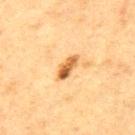* notes: no biopsy performed (imaged during a skin exam)
* lesion diameter: ~3.5 mm (longest diameter)
* body site: the back
* acquisition: total-body-photography crop, ~15 mm field of view
* patient: male, aged around 75
* lighting: cross-polarized illumination